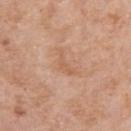Findings:
* lesion size: ≈3.5 mm
* anatomic site: the right upper arm
* patient: female, aged approximately 70
* image source: ~15 mm crop, total-body skin-cancer survey
* tile lighting: white-light illumination